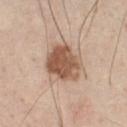The lesion was photographed on a routine skin check and not biopsied; there is no pathology result. Automated tile analysis of the lesion measured a shape eccentricity near 0.5 and a shape-asymmetry score of about 0.25 (0 = symmetric). It also reported a lesion color around L≈55 a*≈19 b*≈30 in CIELAB, roughly 15 lightness units darker than nearby skin, and a normalized lesion–skin contrast near 10. The analysis additionally found a border-irregularity rating of about 2.5/10, a color-variation rating of about 5/10, and peripheral color asymmetry of about 1.5. Measured at roughly 5 mm in maximum diameter. From the chest. The patient is a male about 45 years old. Captured under white-light illumination. A roughly 15 mm field-of-view crop from a total-body skin photograph.